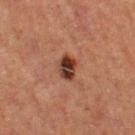<case>
<biopsy_status>not biopsied; imaged during a skin examination</biopsy_status>
<lesion_size>
  <long_diameter_mm_approx>3.0</long_diameter_mm_approx>
</lesion_size>
<image>
  <source>total-body photography crop</source>
  <field_of_view_mm>15</field_of_view_mm>
</image>
<patient>
  <sex>female</sex>
  <age_approx>40</age_approx>
</patient>
<lighting>cross-polarized</lighting>
<automated_metrics>
  <cielab_L>31</cielab_L>
  <cielab_a>20</cielab_a>
  <cielab_b>24</cielab_b>
  <vs_skin_darker_L>13.0</vs_skin_darker_L>
  <vs_skin_contrast_norm>12.0</vs_skin_contrast_norm>
  <color_variation_0_10>8.5</color_variation_0_10>
</automated_metrics>
<site>left thigh</site>
</case>